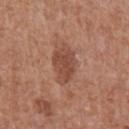Part of a total-body skin-imaging series; this lesion was reviewed on a skin check and was not flagged for biopsy. From the abdomen. Automated tile analysis of the lesion measured a lesion–skin lightness drop of about 10 and a lesion-to-skin contrast of about 7 (normalized; higher = more distinct). And it measured an automated nevus-likeness rating near 45 out of 100 and lesion-presence confidence of about 100/100. The subject is a male in their mid- to late 60s. A 15 mm close-up tile from a total-body photography series done for melanoma screening. Imaged with white-light lighting.